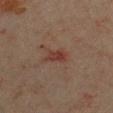Q: Was this lesion biopsied?
A: no biopsy performed (imaged during a skin exam)
Q: How was this image acquired?
A: ~15 mm tile from a whole-body skin photo
Q: Who is the patient?
A: female, approximately 40 years of age
Q: What is the anatomic site?
A: the chest